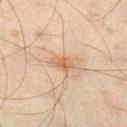location = the leg; tile lighting = cross-polarized illumination; patient = male, aged approximately 45; imaging modality = total-body-photography crop, ~15 mm field of view; diameter = about 3 mm.